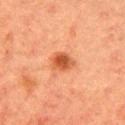Clinical impression:
No biopsy was performed on this lesion — it was imaged during a full skin examination and was not determined to be concerning.
Context:
About 3 mm across. An algorithmic analysis of the crop reported border irregularity of about 2 on a 0–10 scale, internal color variation of about 4 on a 0–10 scale, and radial color variation of about 1.5. And it measured a classifier nevus-likeness of about 95/100 and a detector confidence of about 100 out of 100 that the crop contains a lesion. A close-up tile cropped from a whole-body skin photograph, about 15 mm across. From the right upper arm. Captured under cross-polarized illumination. The subject is a female roughly 55 years of age.